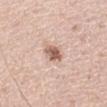Q: Is there a histopathology result?
A: catalogued during a skin exam; not biopsied
Q: Where on the body is the lesion?
A: the right upper arm
Q: What are the patient's age and sex?
A: male, in their mid-40s
Q: What kind of image is this?
A: total-body-photography crop, ~15 mm field of view